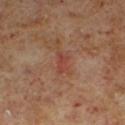Findings:
* follow-up · no biopsy performed (imaged during a skin exam)
* TBP lesion metrics · an area of roughly 3 mm², a shape eccentricity near 0.85, and a symmetry-axis asymmetry near 0.35; a mean CIELAB color near L≈40 a*≈24 b*≈27 and a lesion–skin lightness drop of about 6; a border-irregularity rating of about 3.5/10, a color-variation rating of about 2/10, and radial color variation of about 1
* patient · male, aged approximately 60
* anatomic site · the leg
* image · total-body-photography crop, ~15 mm field of view